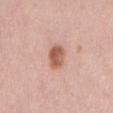Recorded during total-body skin imaging; not selected for excision or biopsy. From the mid back. A close-up tile cropped from a whole-body skin photograph, about 15 mm across. Automated tile analysis of the lesion measured a border-irregularity rating of about 2/10 and a color-variation rating of about 3.5/10. The software also gave a classifier nevus-likeness of about 95/100. The recorded lesion diameter is about 3.5 mm. This is a white-light tile. A male subject, approximately 50 years of age.